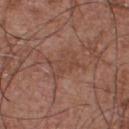<tbp_lesion>
  <biopsy_status>not biopsied; imaged during a skin examination</biopsy_status>
  <patient>
    <sex>male</sex>
    <age_approx>55</age_approx>
  </patient>
  <lighting>white-light</lighting>
  <image>
    <source>total-body photography crop</source>
    <field_of_view_mm>15</field_of_view_mm>
  </image>
  <lesion_size>
    <long_diameter_mm_approx>4.0</long_diameter_mm_approx>
  </lesion_size>
  <site>chest</site>
</tbp_lesion>